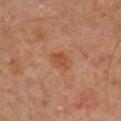| key | value |
|---|---|
| follow-up | catalogued during a skin exam; not biopsied |
| lighting | cross-polarized |
| anatomic site | the right lower leg |
| size | ≈2.5 mm |
| subject | male, aged 58–62 |
| image source | ~15 mm crop, total-body skin-cancer survey |